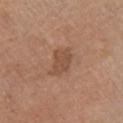{"biopsy_status": "not biopsied; imaged during a skin examination", "site": "left leg", "lighting": "white-light", "image": {"source": "total-body photography crop", "field_of_view_mm": 15}, "patient": {"sex": "female", "age_approx": 65}}